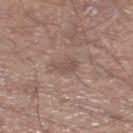Impression: This lesion was catalogued during total-body skin photography and was not selected for biopsy. Image and clinical context: Longest diameter approximately 2.5 mm. Imaged with white-light lighting. The lesion is on the left lower leg. Cropped from a total-body skin-imaging series; the visible field is about 15 mm. A male patient aged 63–67. An algorithmic analysis of the crop reported an area of roughly 4 mm², an eccentricity of roughly 0.7, and a symmetry-axis asymmetry near 0.3. It also reported an average lesion color of about L≈51 a*≈16 b*≈22 (CIELAB) and about 7 CIELAB-L* units darker than the surrounding skin.